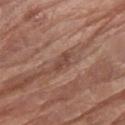imaging modality: 15 mm crop, total-body photography
anatomic site: the right forearm
lighting: white-light
patient: female, aged approximately 75
size: ≈2.5 mm
image-analysis metrics: a mean CIELAB color near L≈45 a*≈21 b*≈26, about 9 CIELAB-L* units darker than the surrounding skin, and a lesion-to-skin contrast of about 7 (normalized; higher = more distinct); a border-irregularity index near 3/10 and a color-variation rating of about 0/10